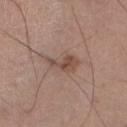Clinical impression:
Imaged during a routine full-body skin examination; the lesion was not biopsied and no histopathology is available.
Acquisition and patient details:
Imaged with white-light lighting. A male patient approximately 55 years of age. A 15 mm crop from a total-body photograph taken for skin-cancer surveillance. Located on the right lower leg. Longest diameter approximately 4.5 mm. Automated tile analysis of the lesion measured a lesion color around L≈49 a*≈18 b*≈25 in CIELAB, roughly 9 lightness units darker than nearby skin, and a normalized border contrast of about 6.5. It also reported a border-irregularity index near 4.5/10 and radial color variation of about 1.5. The analysis additionally found a classifier nevus-likeness of about 20/100 and a detector confidence of about 100 out of 100 that the crop contains a lesion.